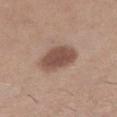Case summary:
• anatomic site: the right lower leg
• lesion diameter: about 4.5 mm
• subject: female, roughly 45 years of age
• tile lighting: white-light illumination
• image: 15 mm crop, total-body photography